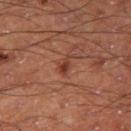Part of a total-body skin-imaging series; this lesion was reviewed on a skin check and was not flagged for biopsy.
A male patient about 60 years old.
The lesion is on the right thigh.
A 15 mm close-up tile from a total-body photography series done for melanoma screening.